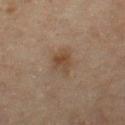biopsy_status: not biopsied; imaged during a skin examination
lighting: cross-polarized
patient:
  sex: female
  age_approx: 55
site: leg
lesion_size:
  long_diameter_mm_approx: 3.0
image:
  source: total-body photography crop
  field_of_view_mm: 15